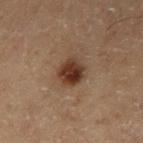Notes:
• imaging modality — total-body-photography crop, ~15 mm field of view
• image-analysis metrics — a mean CIELAB color near L≈27 a*≈15 b*≈22 and a normalized lesion–skin contrast near 11
• subject — male, aged 68 to 72
• location — the left thigh
• illumination — cross-polarized illumination